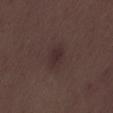Q: Is there a histopathology result?
A: catalogued during a skin exam; not biopsied
Q: What are the patient's age and sex?
A: male, aged around 50
Q: How was this image acquired?
A: total-body-photography crop, ~15 mm field of view